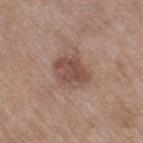The lesion was photographed on a routine skin check and not biopsied; there is no pathology result. A female subject, in their mid-50s. The lesion is on the right thigh. This is a white-light tile. Cropped from a whole-body photographic skin survey; the tile spans about 15 mm.